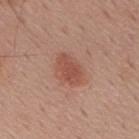Case summary:
- follow-up · imaged on a skin check; not biopsied
- acquisition · ~15 mm tile from a whole-body skin photo
- patient · male, approximately 60 years of age
- anatomic site · the upper back